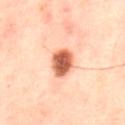| key | value |
|---|---|
| workup | total-body-photography surveillance lesion; no biopsy |
| patient | male, aged around 60 |
| diameter | ≈3.5 mm |
| illumination | cross-polarized |
| image source | total-body-photography crop, ~15 mm field of view |
| image-analysis metrics | an area of roughly 7.5 mm², an eccentricity of roughly 0.55, and a symmetry-axis asymmetry near 0.2; a border-irregularity rating of about 1.5/10, internal color variation of about 3.5 on a 0–10 scale, and a peripheral color-asymmetry measure near 1 |
| body site | the abdomen |